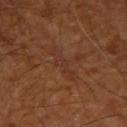notes: no biopsy performed (imaged during a skin exam); acquisition: total-body-photography crop, ~15 mm field of view; tile lighting: cross-polarized illumination; size: about 4 mm; site: the right upper arm; patient: aged 63 to 67.